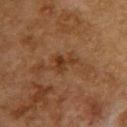From the upper back. The subject is a female about 60 years old. The tile uses cross-polarized illumination. A 15 mm close-up tile from a total-body photography series done for melanoma screening.